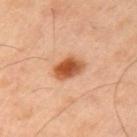{
  "site": "left upper arm",
  "image": {
    "source": "total-body photography crop",
    "field_of_view_mm": 15
  },
  "patient": {
    "sex": "male",
    "age_approx": 45
  }
}